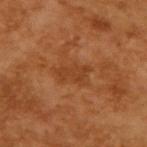- workup — imaged on a skin check; not biopsied
- image — ~15 mm crop, total-body skin-cancer survey
- automated metrics — a shape eccentricity near 0.85 and a shape-asymmetry score of about 0.35 (0 = symmetric); a lesion color around L≈40 a*≈25 b*≈38 in CIELAB, about 6 CIELAB-L* units darker than the surrounding skin, and a normalized lesion–skin contrast near 5.5; border irregularity of about 4.5 on a 0–10 scale, internal color variation of about 1.5 on a 0–10 scale, and a peripheral color-asymmetry measure near 0.5
- tile lighting — cross-polarized
- size — ~4 mm (longest diameter)
- subject — male, in their mid- to late 60s